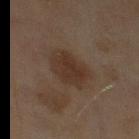follow-up: imaged on a skin check; not biopsied
site: the left thigh
size: ~4.5 mm (longest diameter)
patient: female, in their 60s
image: total-body-photography crop, ~15 mm field of view
TBP lesion metrics: an area of roughly 13 mm², an outline eccentricity of about 0.65 (0 = round, 1 = elongated), and a symmetry-axis asymmetry near 0.2; a lesion color around L≈29 a*≈14 b*≈22 in CIELAB; border irregularity of about 2 on a 0–10 scale, a within-lesion color-variation index near 2.5/10, and peripheral color asymmetry of about 1; a lesion-detection confidence of about 100/100
lighting: cross-polarized